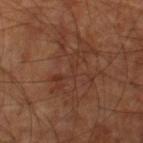follow-up: no biopsy performed (imaged during a skin exam) | image source: ~15 mm tile from a whole-body skin photo | subject: male, about 60 years old | body site: the right thigh | image-analysis metrics: a footprint of about 9.5 mm², a shape eccentricity near 0.95, and a shape-asymmetry score of about 0.6 (0 = symmetric); an automated nevus-likeness rating near 0 out of 100 and a detector confidence of about 80 out of 100 that the crop contains a lesion | lesion diameter: ~6.5 mm (longest diameter).